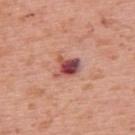No biopsy was performed on this lesion — it was imaged during a full skin examination and was not determined to be concerning.